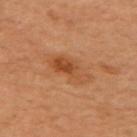Part of a total-body skin-imaging series; this lesion was reviewed on a skin check and was not flagged for biopsy. Approximately 4 mm at its widest. Automated image analysis of the tile measured a mean CIELAB color near L≈42 a*≈23 b*≈34, a lesion–skin lightness drop of about 8, and a normalized lesion–skin contrast near 7. And it measured border irregularity of about 4 on a 0–10 scale. This image is a 15 mm lesion crop taken from a total-body photograph. This is a cross-polarized tile. A female subject, aged 58–62. Located on the right arm.